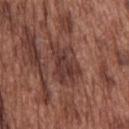A 15 mm close-up extracted from a 3D total-body photography capture. The tile uses white-light illumination. An algorithmic analysis of the crop reported a lesion area of about 14 mm² and a symmetry-axis asymmetry near 0.3. The analysis additionally found an average lesion color of about L≈37 a*≈22 b*≈23 (CIELAB), roughly 9 lightness units darker than nearby skin, and a lesion-to-skin contrast of about 8 (normalized; higher = more distinct). The software also gave a border-irregularity rating of about 3.5/10 and a color-variation rating of about 4/10. The subject is a male aged around 75. Approximately 5.5 mm at its widest. From the upper back.